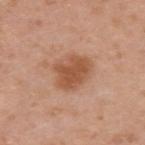| field | value |
|---|---|
| notes | imaged on a skin check; not biopsied |
| location | the upper back |
| size | ≈4.5 mm |
| automated lesion analysis | an average lesion color of about L≈53 a*≈23 b*≈33 (CIELAB), roughly 11 lightness units darker than nearby skin, and a normalized lesion–skin contrast near 8; border irregularity of about 2.5 on a 0–10 scale and radial color variation of about 1 |
| tile lighting | white-light illumination |
| acquisition | total-body-photography crop, ~15 mm field of view |
| subject | male, about 35 years old |